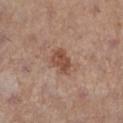Notes:
- biopsy status · catalogued during a skin exam; not biopsied
- subject · female, in their mid-40s
- anatomic site · the leg
- lighting · white-light
- image · ~15 mm crop, total-body skin-cancer survey